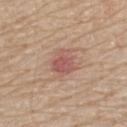Captured during whole-body skin photography for melanoma surveillance; the lesion was not biopsied. The lesion is located on the back. About 3 mm across. Imaged with white-light lighting. A male subject in their 80s. A 15 mm crop from a total-body photograph taken for skin-cancer surveillance.